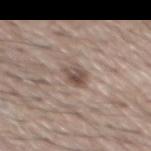Recorded during total-body skin imaging; not selected for excision or biopsy.
The total-body-photography lesion software estimated border irregularity of about 2.5 on a 0–10 scale, internal color variation of about 6 on a 0–10 scale, and radial color variation of about 2.
From the mid back.
A male subject roughly 65 years of age.
A lesion tile, about 15 mm wide, cut from a 3D total-body photograph.